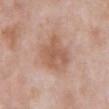<case>
  <biopsy_status>not biopsied; imaged during a skin examination</biopsy_status>
  <lesion_size>
    <long_diameter_mm_approx>4.5</long_diameter_mm_approx>
  </lesion_size>
  <image>
    <source>total-body photography crop</source>
    <field_of_view_mm>15</field_of_view_mm>
  </image>
  <lighting>white-light</lighting>
  <automated_metrics>
    <area_mm2_approx>15.0</area_mm2_approx>
    <shape_asymmetry>0.25</shape_asymmetry>
  </automated_metrics>
  <site>abdomen</site>
  <patient>
    <sex>male</sex>
    <age_approx>55</age_approx>
  </patient>
</case>